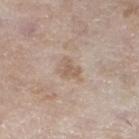Q: Was this lesion biopsied?
A: no biopsy performed (imaged during a skin exam)
Q: Patient demographics?
A: female, in their mid-70s
Q: What kind of image is this?
A: total-body-photography crop, ~15 mm field of view
Q: How large is the lesion?
A: about 3 mm
Q: Lesion location?
A: the left lower leg
Q: What lighting was used for the tile?
A: white-light illumination